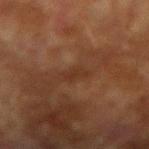  biopsy_status: not biopsied; imaged during a skin examination
  site: right upper arm
  lesion_size:
    long_diameter_mm_approx: 2.5
  lighting: cross-polarized
  image:
    source: total-body photography crop
    field_of_view_mm: 15
  patient:
    sex: male
    age_approx: 75